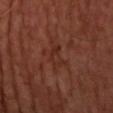biopsy_status: not biopsied; imaged during a skin examination
image:
  source: total-body photography crop
  field_of_view_mm: 15
patient:
  sex: male
  age_approx: 65
lesion_size:
  long_diameter_mm_approx: 3.0
site: head or neck
automated_metrics:
  shape_asymmetry: 0.4
  cielab_L: 28
  cielab_a: 23
  cielab_b: 25
  vs_skin_contrast_norm: 5.0
  nevus_likeness_0_100: 0
lighting: cross-polarized